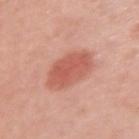No biopsy was performed on this lesion — it was imaged during a full skin examination and was not determined to be concerning. The lesion is located on the left upper arm. A female patient aged 38 to 42. Cropped from a total-body skin-imaging series; the visible field is about 15 mm.